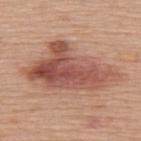Imaged during a routine full-body skin examination; the lesion was not biopsied and no histopathology is available.
A 15 mm crop from a total-body photograph taken for skin-cancer surveillance.
Located on the upper back.
A female subject in their 60s.
The tile uses white-light illumination.
Measured at roughly 11 mm in maximum diameter.